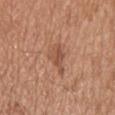Acquisition and patient details:
The subject is a male about 65 years old. On the head or neck. The lesion's longest dimension is about 4.5 mm. Cropped from a whole-body photographic skin survey; the tile spans about 15 mm. The tile uses white-light illumination.